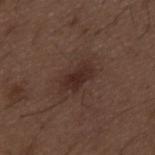biopsy_status: not biopsied; imaged during a skin examination
lesion_size:
  long_diameter_mm_approx: 4.5
site: mid back
image:
  source: total-body photography crop
  field_of_view_mm: 15
patient:
  sex: male
  age_approx: 50